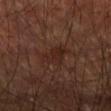biopsy status: no biopsy performed (imaged during a skin exam) | TBP lesion metrics: a footprint of about 5 mm² and a symmetry-axis asymmetry near 0.45; a mean CIELAB color near L≈23 a*≈19 b*≈22 and a lesion-to-skin contrast of about 6.5 (normalized; higher = more distinct) | patient: male, aged around 60 | location: the left upper arm | acquisition: 15 mm crop, total-body photography | lesion diameter: ~3 mm (longest diameter) | tile lighting: cross-polarized.